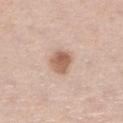This lesion was catalogued during total-body skin photography and was not selected for biopsy.
The patient is a female aged around 65.
Located on the leg.
Longest diameter approximately 3.5 mm.
The tile uses white-light illumination.
Automated image analysis of the tile measured a shape eccentricity near 0.55 and two-axis asymmetry of about 0.25.
A 15 mm close-up tile from a total-body photography series done for melanoma screening.